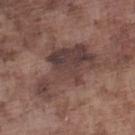Measured at roughly 9 mm in maximum diameter.
A 15 mm close-up extracted from a 3D total-body photography capture.
The lesion is located on the left lower leg.
A male patient, roughly 75 years of age.